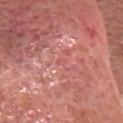Recorded during total-body skin imaging; not selected for excision or biopsy. An algorithmic analysis of the crop reported an area of roughly 1 mm², an outline eccentricity of about 0.8 (0 = round, 1 = elongated), and a shape-asymmetry score of about 0.45 (0 = symmetric). The software also gave a border-irregularity rating of about 3.5/10 and radial color variation of about 0. The software also gave an automated nevus-likeness rating near 0 out of 100. The recorded lesion diameter is about 1 mm. A 15 mm close-up extracted from a 3D total-body photography capture. Imaged with white-light lighting. Located on the head or neck. A female subject aged 48–52.